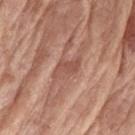Clinical impression:
The lesion was photographed on a routine skin check and not biopsied; there is no pathology result.
Background:
The patient is a female roughly 75 years of age. A 15 mm close-up extracted from a 3D total-body photography capture. On the left upper arm. Approximately 3.5 mm at its widest. This is a white-light tile.